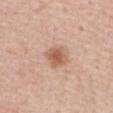This lesion was catalogued during total-body skin photography and was not selected for biopsy.
This is a white-light tile.
About 3 mm across.
Automated tile analysis of the lesion measured a nevus-likeness score of about 85/100 and lesion-presence confidence of about 100/100.
The lesion is on the abdomen.
A male patient, aged 73–77.
A 15 mm close-up extracted from a 3D total-body photography capture.